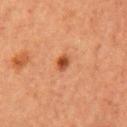Assessment:
No biopsy was performed on this lesion — it was imaged during a full skin examination and was not determined to be concerning.